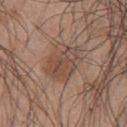Q: Is there a histopathology result?
A: imaged on a skin check; not biopsied
Q: Who is the patient?
A: male, aged 43–47
Q: How was the tile lit?
A: white-light
Q: Lesion size?
A: ~5 mm (longest diameter)
Q: What kind of image is this?
A: ~15 mm tile from a whole-body skin photo
Q: Where on the body is the lesion?
A: the chest